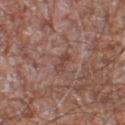tile lighting — white-light
anatomic site — the left lower leg
patient — male, aged approximately 60
size — about 2.5 mm
image — ~15 mm tile from a whole-body skin photo